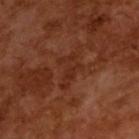Clinical impression: Part of a total-body skin-imaging series; this lesion was reviewed on a skin check and was not flagged for biopsy. Acquisition and patient details: About 4 mm across. The tile uses cross-polarized illumination. This image is a 15 mm lesion crop taken from a total-body photograph. A male patient, in their mid- to late 60s.